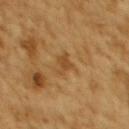Q: Was this lesion biopsied?
A: no biopsy performed (imaged during a skin exam)
Q: Lesion size?
A: ~3 mm (longest diameter)
Q: How was the tile lit?
A: cross-polarized illumination
Q: What are the patient's age and sex?
A: female, in their mid-50s
Q: Where on the body is the lesion?
A: the upper back
Q: How was this image acquired?
A: ~15 mm tile from a whole-body skin photo